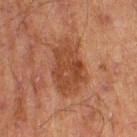Recorded during total-body skin imaging; not selected for excision or biopsy. A close-up tile cropped from a whole-body skin photograph, about 15 mm across. The lesion is located on the right thigh. A male subject roughly 70 years of age. This is a cross-polarized tile. Automated image analysis of the tile measured an eccentricity of roughly 0.8 and a symmetry-axis asymmetry near 0.25. And it measured a lesion color around L≈37 a*≈21 b*≈29 in CIELAB, about 8 CIELAB-L* units darker than the surrounding skin, and a normalized lesion–skin contrast near 7. The analysis additionally found border irregularity of about 3 on a 0–10 scale, a within-lesion color-variation index near 3/10, and radial color variation of about 1. The software also gave an automated nevus-likeness rating near 20 out of 100 and lesion-presence confidence of about 100/100. About 6 mm across.